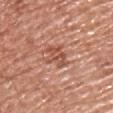follow-up=imaged on a skin check; not biopsied
lesion diameter=~3.5 mm (longest diameter)
patient=male, roughly 70 years of age
site=the chest
image source=~15 mm crop, total-body skin-cancer survey